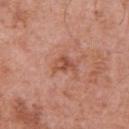notes: catalogued during a skin exam; not biopsied
acquisition: ~15 mm tile from a whole-body skin photo
tile lighting: white-light
anatomic site: the chest
patient: male, aged approximately 70